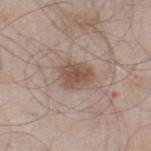image-analysis metrics = an area of roughly 8.5 mm², an eccentricity of roughly 0.55, and two-axis asymmetry of about 0.25
illumination = white-light illumination
site = the right thigh
lesion diameter = ~3.5 mm (longest diameter)
patient = male, aged approximately 60
image = 15 mm crop, total-body photography